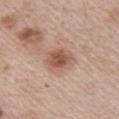  image:
    source: total-body photography crop
    field_of_view_mm: 15
  site: chest
  lesion_size:
    long_diameter_mm_approx: 3.5
  lighting: white-light
  patient:
    sex: male
    age_approx: 65
  automated_metrics:
    nevus_likeness_0_100: 80
    lesion_detection_confidence_0_100: 100